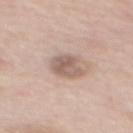Q: Was a biopsy performed?
A: imaged on a skin check; not biopsied
Q: What is the imaging modality?
A: total-body-photography crop, ~15 mm field of view
Q: Where on the body is the lesion?
A: the back
Q: How was the tile lit?
A: white-light
Q: What are the patient's age and sex?
A: female, about 65 years old
Q: What did automated image analysis measure?
A: an area of roughly 7.5 mm² and two-axis asymmetry of about 0.25; an average lesion color of about L≈59 a*≈16 b*≈24 (CIELAB) and about 11 CIELAB-L* units darker than the surrounding skin; border irregularity of about 2.5 on a 0–10 scale, internal color variation of about 4 on a 0–10 scale, and peripheral color asymmetry of about 1.5; a classifier nevus-likeness of about 5/100 and lesion-presence confidence of about 100/100